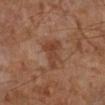Assessment:
Recorded during total-body skin imaging; not selected for excision or biopsy.
Context:
A male subject, in their 60s. This is a cross-polarized tile. Cropped from a total-body skin-imaging series; the visible field is about 15 mm. The lesion is located on the left lower leg.